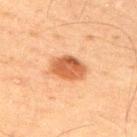{"biopsy_status": "not biopsied; imaged during a skin examination", "lesion_size": {"long_diameter_mm_approx": 4.0}, "image": {"source": "total-body photography crop", "field_of_view_mm": 15}, "lighting": "cross-polarized", "patient": {"sex": "male", "age_approx": 45}, "site": "arm"}